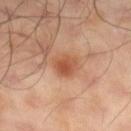* follow-up · catalogued during a skin exam; not biopsied
* size · about 3 mm
* imaging modality · ~15 mm tile from a whole-body skin photo
* image-analysis metrics · an average lesion color of about L≈50 a*≈24 b*≈33 (CIELAB), roughly 9 lightness units darker than nearby skin, and a normalized border contrast of about 7.5; radial color variation of about 1; a nevus-likeness score of about 65/100
* lighting · cross-polarized
* subject · male, aged around 65
* site · the right lower leg